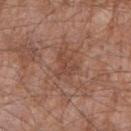follow-up = catalogued during a skin exam; not biopsied
size = ≈3 mm
imaging modality = ~15 mm tile from a whole-body skin photo
site = the right forearm
automated metrics = an average lesion color of about L≈45 a*≈22 b*≈27 (CIELAB), about 6 CIELAB-L* units darker than the surrounding skin, and a normalized lesion–skin contrast near 5
lighting = white-light
patient = male, aged 53 to 57